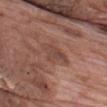A male patient in their 70s. A 15 mm close-up extracted from a 3D total-body photography capture. The lesion is on the upper back. Captured under white-light illumination.